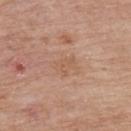Clinical impression: Captured during whole-body skin photography for melanoma surveillance; the lesion was not biopsied. Image and clinical context: A region of skin cropped from a whole-body photographic capture, roughly 15 mm wide. The recorded lesion diameter is about 2.5 mm. The lesion is on the upper back. A male subject aged 73–77.